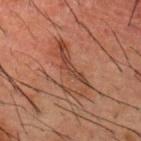Recorded during total-body skin imaging; not selected for excision or biopsy.
Cropped from a total-body skin-imaging series; the visible field is about 15 mm.
The subject is a male roughly 50 years of age.
From the back.
This is a cross-polarized tile.